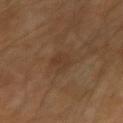biopsy_status: not biopsied; imaged during a skin examination
lighting: cross-polarized
site: right upper arm
patient:
  sex: male
  age_approx: 60
image:
  source: total-body photography crop
  field_of_view_mm: 15